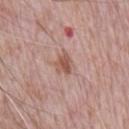Impression: The lesion was tiled from a total-body skin photograph and was not biopsied. Background: From the chest. Measured at roughly 2.5 mm in maximum diameter. A 15 mm close-up tile from a total-body photography series done for melanoma screening. A male patient, about 70 years old.